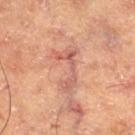This lesion was catalogued during total-body skin photography and was not selected for biopsy. A 15 mm close-up tile from a total-body photography series done for melanoma screening. Located on the right thigh. A male patient, approximately 65 years of age.